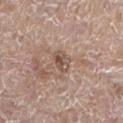Q: Who is the patient?
A: female, aged 83 or older
Q: What did automated image analysis measure?
A: an area of roughly 4.5 mm², an outline eccentricity of about 0.6 (0 = round, 1 = elongated), and a shape-asymmetry score of about 0.2 (0 = symmetric); a mean CIELAB color near L≈52 a*≈17 b*≈25, roughly 10 lightness units darker than nearby skin, and a normalized border contrast of about 7; a nevus-likeness score of about 0/100 and lesion-presence confidence of about 100/100
Q: How was the tile lit?
A: white-light illumination
Q: Where on the body is the lesion?
A: the left lower leg
Q: Lesion size?
A: about 2.5 mm
Q: What is the imaging modality?
A: 15 mm crop, total-body photography